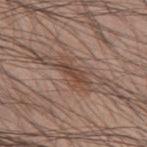Q: What are the patient's age and sex?
A: male, in their 60s
Q: How large is the lesion?
A: about 3.5 mm
Q: Where on the body is the lesion?
A: the mid back
Q: What is the imaging modality?
A: 15 mm crop, total-body photography